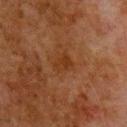automated_metrics:
  eccentricity: 0.65
  shape_asymmetry: 0.35
  cielab_L: 27
  cielab_a: 20
  cielab_b: 29
  vs_skin_darker_L: 5.0
  vs_skin_contrast_norm: 6.0
  nevus_likeness_0_100: 0
lesion_size:
  long_diameter_mm_approx: 2.5
lighting: cross-polarized
site: upper back
image:
  source: total-body photography crop
  field_of_view_mm: 15
patient:
  sex: male
  age_approx: 80